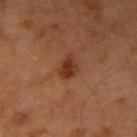The lesion was tiled from a total-body skin photograph and was not biopsied. A male subject, in their mid- to late 60s. A lesion tile, about 15 mm wide, cut from a 3D total-body photograph. The total-body-photography lesion software estimated a footprint of about 4.5 mm², an outline eccentricity of about 0.7 (0 = round, 1 = elongated), and a shape-asymmetry score of about 0.3 (0 = symmetric). The software also gave a mean CIELAB color near L≈34 a*≈25 b*≈32 and a normalized lesion–skin contrast near 8.5. And it measured a border-irregularity rating of about 3/10. The lesion is located on the right upper arm. The recorded lesion diameter is about 3 mm.